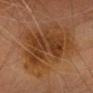Impression:
The lesion was photographed on a routine skin check and not biopsied; there is no pathology result.
Acquisition and patient details:
A 15 mm close-up extracted from a 3D total-body photography capture. From the head or neck. The patient is a female aged 58–62. An algorithmic analysis of the crop reported an area of roughly 50 mm² and an outline eccentricity of about 0.6 (0 = round, 1 = elongated). It also reported an average lesion color of about L≈35 a*≈19 b*≈31 (CIELAB), about 8 CIELAB-L* units darker than the surrounding skin, and a normalized border contrast of about 8. And it measured border irregularity of about 5 on a 0–10 scale and a color-variation rating of about 5/10. And it measured a nevus-likeness score of about 20/100 and a detector confidence of about 100 out of 100 that the crop contains a lesion.